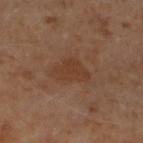Case summary:
– image source — 15 mm crop, total-body photography
– patient — male, aged around 60
– tile lighting — cross-polarized
– automated metrics — an area of roughly 7.5 mm² and an outline eccentricity of about 0.75 (0 = round, 1 = elongated); a lesion color around L≈35 a*≈18 b*≈27 in CIELAB, a lesion–skin lightness drop of about 5, and a normalized border contrast of about 6
– body site — the left lower leg
– lesion size — about 4 mm